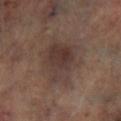Recorded during total-body skin imaging; not selected for excision or biopsy.
Measured at roughly 6 mm in maximum diameter.
The lesion-visualizer software estimated a lesion color around L≈34 a*≈14 b*≈19 in CIELAB, about 7 CIELAB-L* units darker than the surrounding skin, and a lesion-to-skin contrast of about 7 (normalized; higher = more distinct).
The tile uses cross-polarized illumination.
A male patient roughly 65 years of age.
Cropped from a whole-body photographic skin survey; the tile spans about 15 mm.
The lesion is on the left lower leg.